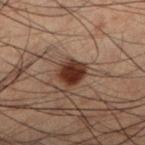Assessment: Imaged during a routine full-body skin examination; the lesion was not biopsied and no histopathology is available. Context: The patient is a male aged 48 to 52. Captured under cross-polarized illumination. The lesion's longest dimension is about 3 mm. The lesion is located on the left lower leg. A close-up tile cropped from a whole-body skin photograph, about 15 mm across. An algorithmic analysis of the crop reported a lesion area of about 6.5 mm² and two-axis asymmetry of about 0.2. And it measured an average lesion color of about L≈24 a*≈17 b*≈21 (CIELAB), roughly 13 lightness units darker than nearby skin, and a normalized lesion–skin contrast near 13.5.